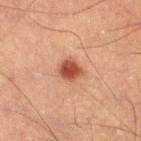Imaged during a routine full-body skin examination; the lesion was not biopsied and no histopathology is available.
Imaged with cross-polarized lighting.
The subject is a male aged 53–57.
An algorithmic analysis of the crop reported a footprint of about 5 mm², an eccentricity of roughly 0.5, and a shape-asymmetry score of about 0.25 (0 = symmetric). The software also gave a lesion color around L≈44 a*≈25 b*≈29 in CIELAB and a lesion–skin lightness drop of about 14. It also reported a border-irregularity rating of about 2/10, internal color variation of about 4 on a 0–10 scale, and peripheral color asymmetry of about 1. The software also gave a nevus-likeness score of about 100/100.
The lesion is located on the leg.
A 15 mm crop from a total-body photograph taken for skin-cancer surveillance.